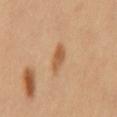Q: Was a biopsy performed?
A: total-body-photography surveillance lesion; no biopsy
Q: How was this image acquired?
A: ~15 mm crop, total-body skin-cancer survey
Q: What are the patient's age and sex?
A: female, aged around 50